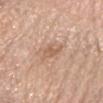Acquisition and patient details: A 15 mm close-up tile from a total-body photography series done for melanoma screening. The lesion's longest dimension is about 3 mm. The lesion is on the chest. A female subject roughly 65 years of age.